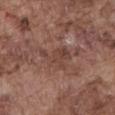Impression:
This lesion was catalogued during total-body skin photography and was not selected for biopsy.
Context:
The tile uses white-light illumination. The lesion is on the abdomen. A region of skin cropped from a whole-body photographic capture, roughly 15 mm wide. The patient is a male approximately 75 years of age. About 4 mm across.